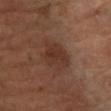Context:
The lesion is on the left lower leg. A 15 mm close-up tile from a total-body photography series done for melanoma screening. The tile uses cross-polarized illumination. Measured at roughly 4 mm in maximum diameter. The patient is a female aged approximately 65.